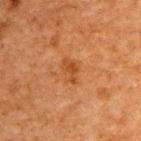Impression:
The lesion was photographed on a routine skin check and not biopsied; there is no pathology result.
Context:
The patient is a male aged 48 to 52. The lesion is on the upper back. A region of skin cropped from a whole-body photographic capture, roughly 15 mm wide.